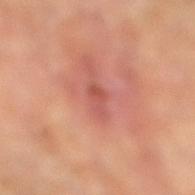Notes:
– workup — total-body-photography surveillance lesion; no biopsy
– subject — female, in their mid- to late 60s
– lesion size — about 2.5 mm
– illumination — cross-polarized
– acquisition — total-body-photography crop, ~15 mm field of view
– automated metrics — an average lesion color of about L≈52 a*≈30 b*≈28 (CIELAB) and a lesion–skin lightness drop of about 8; a classifier nevus-likeness of about 0/100 and a lesion-detection confidence of about 95/100
– site — the left leg